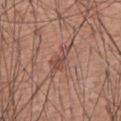• notes: total-body-photography surveillance lesion; no biopsy
• diameter: about 4 mm
• subject: male, aged 58–62
• imaging modality: total-body-photography crop, ~15 mm field of view
• site: the chest